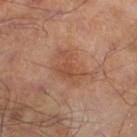Q: How was this image acquired?
A: total-body-photography crop, ~15 mm field of view
Q: What are the patient's age and sex?
A: male, in their mid- to late 60s
Q: Lesion size?
A: ~4.5 mm (longest diameter)
Q: Illumination type?
A: cross-polarized
Q: Lesion location?
A: the left lower leg